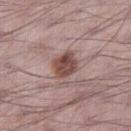biopsy status — imaged on a skin check; not biopsied
image source — ~15 mm tile from a whole-body skin photo
location — the right thigh
lesion size — ~3 mm (longest diameter)
patient — male, aged 68 to 72